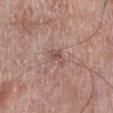Q: What are the patient's age and sex?
A: male, in their mid-70s
Q: What kind of image is this?
A: ~15 mm tile from a whole-body skin photo
Q: Lesion size?
A: ~2.5 mm (longest diameter)
Q: Where on the body is the lesion?
A: the right lower leg
Q: How was the tile lit?
A: white-light illumination
Q: What did automated image analysis measure?
A: a lesion area of about 3 mm² and an eccentricity of roughly 0.8; a lesion color around L≈51 a*≈19 b*≈21 in CIELAB; a nevus-likeness score of about 0/100 and a detector confidence of about 100 out of 100 that the crop contains a lesion